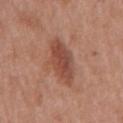Q: Was a biopsy performed?
A: no biopsy performed (imaged during a skin exam)
Q: Illumination type?
A: white-light
Q: What is the anatomic site?
A: the mid back
Q: What kind of image is this?
A: ~15 mm tile from a whole-body skin photo
Q: Lesion size?
A: ~5 mm (longest diameter)
Q: Patient demographics?
A: male, in their 70s
Q: What did automated image analysis measure?
A: a lesion area of about 11 mm² and two-axis asymmetry of about 0.15; roughly 10 lightness units darker than nearby skin and a normalized lesion–skin contrast near 7.5